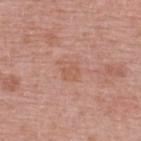Q: Was this lesion biopsied?
A: imaged on a skin check; not biopsied
Q: Automated lesion metrics?
A: a footprint of about 4.5 mm², an eccentricity of roughly 0.55, and a shape-asymmetry score of about 0.35 (0 = symmetric); an average lesion color of about L≈57 a*≈23 b*≈30 (CIELAB), about 6 CIELAB-L* units darker than the surrounding skin, and a normalized border contrast of about 5; a nevus-likeness score of about 0/100
Q: How large is the lesion?
A: ≈2.5 mm
Q: What is the anatomic site?
A: the upper back
Q: What is the imaging modality?
A: total-body-photography crop, ~15 mm field of view
Q: Patient demographics?
A: female, aged 58 to 62
Q: Illumination type?
A: white-light illumination